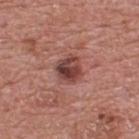This lesion was catalogued during total-body skin photography and was not selected for biopsy. Located on the upper back. The subject is a male approximately 70 years of age. A roughly 15 mm field-of-view crop from a total-body skin photograph. The lesion's longest dimension is about 3 mm. The total-body-photography lesion software estimated a nevus-likeness score of about 70/100 and a detector confidence of about 100 out of 100 that the crop contains a lesion. Captured under white-light illumination.